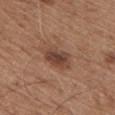Notes:
- notes · imaged on a skin check; not biopsied
- patient · male, aged 63 to 67
- size · ~3.5 mm (longest diameter)
- illumination · white-light
- imaging modality · ~15 mm tile from a whole-body skin photo
- anatomic site · the chest